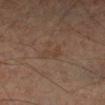The lesion was photographed on a routine skin check and not biopsied; there is no pathology result.
The lesion is on the right lower leg.
A male patient, about 60 years old.
A 15 mm close-up extracted from a 3D total-body photography capture.